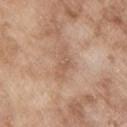Impression: Captured during whole-body skin photography for melanoma surveillance; the lesion was not biopsied. Acquisition and patient details: The recorded lesion diameter is about 4 mm. Located on the upper back. A roughly 15 mm field-of-view crop from a total-body skin photograph. The total-body-photography lesion software estimated an automated nevus-likeness rating near 0 out of 100 and a detector confidence of about 100 out of 100 that the crop contains a lesion. This is a white-light tile. The subject is a male about 65 years old.